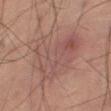Q: What kind of image is this?
A: ~15 mm tile from a whole-body skin photo
Q: Patient demographics?
A: male, aged 58 to 62
Q: What is the anatomic site?
A: the leg
Q: Lesion size?
A: about 8.5 mm